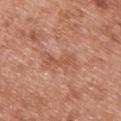Assessment:
The lesion was photographed on a routine skin check and not biopsied; there is no pathology result.
Acquisition and patient details:
A 15 mm close-up tile from a total-body photography series done for melanoma screening. A female patient roughly 40 years of age. The lesion's longest dimension is about 4.5 mm. From the upper back. Captured under white-light illumination.